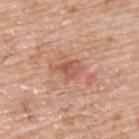No biopsy was performed on this lesion — it was imaged during a full skin examination and was not determined to be concerning. The lesion is located on the back. The lesion's longest dimension is about 3 mm. The total-body-photography lesion software estimated a lesion area of about 4.5 mm², an outline eccentricity of about 0.55 (0 = round, 1 = elongated), and a symmetry-axis asymmetry near 0.35. The software also gave a classifier nevus-likeness of about 0/100 and lesion-presence confidence of about 100/100. A male subject, aged 73–77. A roughly 15 mm field-of-view crop from a total-body skin photograph. The tile uses white-light illumination.